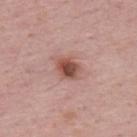  biopsy_status: not biopsied; imaged during a skin examination
  site: upper back
  image:
    source: total-body photography crop
    field_of_view_mm: 15
  lighting: white-light
  lesion_size:
    long_diameter_mm_approx: 3.0
  patient:
    sex: male
    age_approx: 50
  automated_metrics:
    area_mm2_approx: 5.5
    eccentricity: 0.6
    shape_asymmetry: 0.2
    cielab_L: 49
    cielab_a: 24
    cielab_b: 26
    vs_skin_darker_L: 14.0